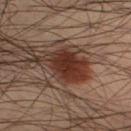  biopsy_status: not biopsied; imaged during a skin examination
  lighting: cross-polarized
  image:
    source: total-body photography crop
    field_of_view_mm: 15
  site: leg
  patient:
    sex: male
    age_approx: 40